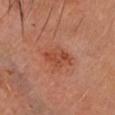Assessment:
Recorded during total-body skin imaging; not selected for excision or biopsy.
Image and clinical context:
A female subject aged 58 to 62. The total-body-photography lesion software estimated a within-lesion color-variation index near 3.5/10. The software also gave a nevus-likeness score of about 35/100. From the head or neck. This is a cross-polarized tile. A lesion tile, about 15 mm wide, cut from a 3D total-body photograph. About 3.5 mm across.